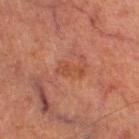site: left thigh
automated_metrics:
  cielab_L: 38
  cielab_a: 23
  cielab_b: 28
  vs_skin_contrast_norm: 5.5
lighting: cross-polarized
image:
  source: total-body photography crop
  field_of_view_mm: 15
patient:
  sex: male
  age_approx: 65